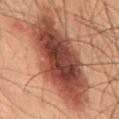illumination = cross-polarized
image-analysis metrics = a lesion area of about 50 mm² and a shape eccentricity near 0.9; a border-irregularity rating of about 3.5/10 and internal color variation of about 6.5 on a 0–10 scale; an automated nevus-likeness rating near 95 out of 100 and a lesion-detection confidence of about 100/100
location = the mid back
acquisition = ~15 mm crop, total-body skin-cancer survey
diameter = ~12.5 mm (longest diameter)
patient = male, aged 48 to 52